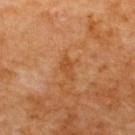| key | value |
|---|---|
| workup | imaged on a skin check; not biopsied |
| patient | female, aged around 65 |
| image-analysis metrics | an average lesion color of about L≈49 a*≈26 b*≈40 (CIELAB) and a normalized border contrast of about 5.5; a border-irregularity rating of about 3/10; a classifier nevus-likeness of about 0/100 |
| body site | the upper back |
| diameter | about 3 mm |
| image source | 15 mm crop, total-body photography |